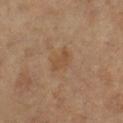Clinical impression:
Part of a total-body skin-imaging series; this lesion was reviewed on a skin check and was not flagged for biopsy.
Background:
The lesion's longest dimension is about 3 mm. On the leg. A female patient aged 68 to 72. Cropped from a whole-body photographic skin survey; the tile spans about 15 mm. This is a cross-polarized tile.